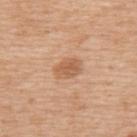Assessment:
Captured during whole-body skin photography for melanoma surveillance; the lesion was not biopsied.
Clinical summary:
A roughly 15 mm field-of-view crop from a total-body skin photograph. The patient is a female aged 53 to 57. Approximately 3 mm at its widest. This is a white-light tile. From the back.